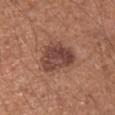Assessment: Part of a total-body skin-imaging series; this lesion was reviewed on a skin check and was not flagged for biopsy. Background: This image is a 15 mm lesion crop taken from a total-body photograph. Captured under white-light illumination. A male subject, in their mid- to late 50s. About 4.5 mm across. On the arm.